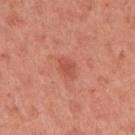{"biopsy_status": "not biopsied; imaged during a skin examination", "patient": {"sex": "female", "age_approx": 50}, "image": {"source": "total-body photography crop", "field_of_view_mm": 15}, "lighting": "white-light", "lesion_size": {"long_diameter_mm_approx": 3.0}, "site": "arm"}